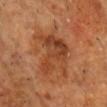Approximately 6.5 mm at its widest. Imaged with cross-polarized lighting. This image is a 15 mm lesion crop taken from a total-body photograph. The lesion is on the head or neck. A male patient, aged approximately 55.